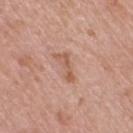The recorded lesion diameter is about 3.5 mm. Automated image analysis of the tile measured about 9 CIELAB-L* units darker than the surrounding skin and a normalized lesion–skin contrast near 6.5. And it measured a detector confidence of about 100 out of 100 that the crop contains a lesion. A lesion tile, about 15 mm wide, cut from a 3D total-body photograph. A female patient, approximately 70 years of age. The lesion is on the right thigh.